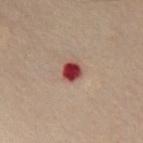<record>
  <site>front of the torso</site>
  <image>
    <source>total-body photography crop</source>
    <field_of_view_mm>15</field_of_view_mm>
  </image>
  <patient>
    <sex>male</sex>
    <age_approx>50</age_approx>
  </patient>
</record>